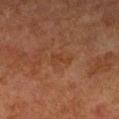{
  "image": {
    "source": "total-body photography crop",
    "field_of_view_mm": 15
  },
  "lighting": "cross-polarized",
  "patient": {
    "sex": "female",
    "age_approx": 60
  },
  "lesion_size": {
    "long_diameter_mm_approx": 3.0
  },
  "site": "right upper arm"
}